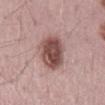Case summary:
• follow-up — no biopsy performed (imaged during a skin exam)
• anatomic site — the mid back
• lesion diameter — about 6 mm
• patient — male, aged 68–72
• tile lighting — white-light
• acquisition — 15 mm crop, total-body photography
• image-analysis metrics — an outline eccentricity of about 0.85 (0 = round, 1 = elongated) and a symmetry-axis asymmetry near 0.15; an average lesion color of about L≈50 a*≈21 b*≈21 (CIELAB), roughly 16 lightness units darker than nearby skin, and a normalized lesion–skin contrast near 10.5; a color-variation rating of about 5.5/10 and peripheral color asymmetry of about 2